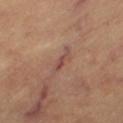biopsy status: total-body-photography surveillance lesion; no biopsy | subject: aged 58–62 | location: the left thigh | image source: total-body-photography crop, ~15 mm field of view.